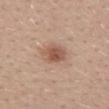biopsy_status: not biopsied; imaged during a skin examination
site: upper back
image:
  source: total-body photography crop
  field_of_view_mm: 15
automated_metrics:
  border_irregularity_0_10: 2.0
  color_variation_0_10: 3.0
  peripheral_color_asymmetry: 1.0
lighting: white-light
patient:
  sex: female
  age_approx: 30